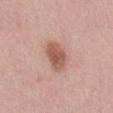Assessment:
No biopsy was performed on this lesion — it was imaged during a full skin examination and was not determined to be concerning.
Image and clinical context:
A male subject aged around 55. A 15 mm close-up extracted from a 3D total-body photography capture. From the mid back.